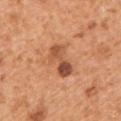workup = total-body-photography surveillance lesion; no biopsy
size = ≈4.5 mm
imaging modality = ~15 mm tile from a whole-body skin photo
lighting = white-light illumination
patient = male, aged 53–57
site = the left upper arm